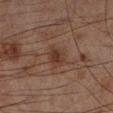Part of a total-body skin-imaging series; this lesion was reviewed on a skin check and was not flagged for biopsy.
A 15 mm close-up extracted from a 3D total-body photography capture.
This is a cross-polarized tile.
A male subject in their mid- to late 60s.
Automated image analysis of the tile measured an area of roughly 5 mm² and an outline eccentricity of about 0.7 (0 = round, 1 = elongated). And it measured a color-variation rating of about 2.5/10 and a peripheral color-asymmetry measure near 1. The analysis additionally found a nevus-likeness score of about 50/100 and a lesion-detection confidence of about 100/100.
On the left lower leg.